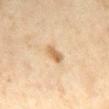Q: Was a biopsy performed?
A: no biopsy performed (imaged during a skin exam)
Q: How was this image acquired?
A: 15 mm crop, total-body photography
Q: Lesion size?
A: ≈2.5 mm
Q: Illumination type?
A: cross-polarized
Q: What are the patient's age and sex?
A: female, aged approximately 60
Q: Where on the body is the lesion?
A: the mid back